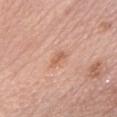Findings:
- biopsy status — catalogued during a skin exam; not biopsied
- acquisition — ~15 mm crop, total-body skin-cancer survey
- subject — female, aged 63 to 67
- body site — the head or neck